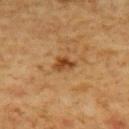Recorded during total-body skin imaging; not selected for excision or biopsy.
Located on the upper back.
A male patient, aged approximately 60.
A region of skin cropped from a whole-body photographic capture, roughly 15 mm wide.
The recorded lesion diameter is about 3 mm.
The tile uses cross-polarized illumination.